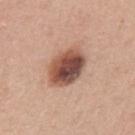patient:
  sex: female
  age_approx: 40
image:
  source: total-body photography crop
  field_of_view_mm: 15
site: left upper arm
lighting: white-light
lesion_size:
  long_diameter_mm_approx: 5.0